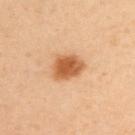The lesion was tiled from a total-body skin photograph and was not biopsied. The lesion's longest dimension is about 4 mm. An algorithmic analysis of the crop reported a mean CIELAB color near L≈57 a*≈25 b*≈40 and a lesion–skin lightness drop of about 15. And it measured a border-irregularity rating of about 2/10, a within-lesion color-variation index near 3/10, and peripheral color asymmetry of about 1. A male patient, in their mid- to late 40s. Located on the upper back. Imaged with cross-polarized lighting. A 15 mm crop from a total-body photograph taken for skin-cancer surveillance.